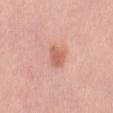Q: Is there a histopathology result?
A: no biopsy performed (imaged during a skin exam)
Q: Illumination type?
A: white-light
Q: How large is the lesion?
A: about 3 mm
Q: Where on the body is the lesion?
A: the mid back
Q: What are the patient's age and sex?
A: female, aged around 55
Q: What is the imaging modality?
A: 15 mm crop, total-body photography